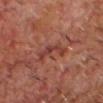No biopsy was performed on this lesion — it was imaged during a full skin examination and was not determined to be concerning. The tile uses cross-polarized illumination. This image is a 15 mm lesion crop taken from a total-body photograph. About 4.5 mm across. The lesion is on the head or neck. The subject is a male aged 63 to 67. An algorithmic analysis of the crop reported a lesion area of about 6 mm², a shape eccentricity near 0.9, and a symmetry-axis asymmetry near 0.6. And it measured a mean CIELAB color near L≈31 a*≈22 b*≈22, a lesion–skin lightness drop of about 6, and a lesion-to-skin contrast of about 6 (normalized; higher = more distinct).